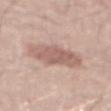{"automated_metrics": {"cielab_L": 60, "cielab_a": 19, "cielab_b": 24, "vs_skin_contrast_norm": 7.0}, "patient": {"sex": "male", "age_approx": 70}, "image": {"source": "total-body photography crop", "field_of_view_mm": 15}, "site": "back", "lesion_size": {"long_diameter_mm_approx": 7.0}}